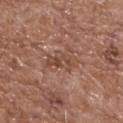Imaged during a routine full-body skin examination; the lesion was not biopsied and no histopathology is available.
The total-body-photography lesion software estimated a footprint of about 7.5 mm² and a shape-asymmetry score of about 0.35 (0 = symmetric). And it measured about 7 CIELAB-L* units darker than the surrounding skin and a normalized lesion–skin contrast near 5. The analysis additionally found a border-irregularity index near 4.5/10 and peripheral color asymmetry of about 1.5. The analysis additionally found a classifier nevus-likeness of about 0/100 and a detector confidence of about 80 out of 100 that the crop contains a lesion.
The lesion is on the upper back.
The recorded lesion diameter is about 4.5 mm.
A close-up tile cropped from a whole-body skin photograph, about 15 mm across.
A male patient, roughly 70 years of age.